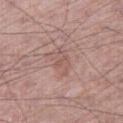Recorded during total-body skin imaging; not selected for excision or biopsy. A male subject roughly 65 years of age. Imaged with white-light lighting. The total-body-photography lesion software estimated an area of roughly 5.5 mm², an outline eccentricity of about 0.8 (0 = round, 1 = elongated), and two-axis asymmetry of about 0.4. The software also gave a nevus-likeness score of about 0/100 and a lesion-detection confidence of about 100/100. Located on the right thigh. This image is a 15 mm lesion crop taken from a total-body photograph.